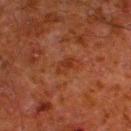Part of a total-body skin-imaging series; this lesion was reviewed on a skin check and was not flagged for biopsy. A region of skin cropped from a whole-body photographic capture, roughly 15 mm wide. Approximately 2.5 mm at its widest. The lesion is on the left lower leg. The lesion-visualizer software estimated a lesion area of about 2.5 mm² and a shape-asymmetry score of about 0.3 (0 = symmetric). The software also gave a lesion color around L≈27 a*≈23 b*≈29 in CIELAB, a lesion–skin lightness drop of about 5, and a lesion-to-skin contrast of about 6 (normalized; higher = more distinct). It also reported a border-irregularity rating of about 2.5/10, internal color variation of about 1.5 on a 0–10 scale, and radial color variation of about 0.5. The analysis additionally found a lesion-detection confidence of about 100/100. The tile uses cross-polarized illumination. A male subject aged around 80.